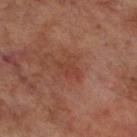{
  "biopsy_status": "not biopsied; imaged during a skin examination",
  "site": "right lower leg",
  "patient": {
    "sex": "male",
    "age_approx": 70
  },
  "image": {
    "source": "total-body photography crop",
    "field_of_view_mm": 15
  }
}